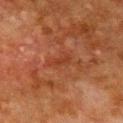Part of a total-body skin-imaging series; this lesion was reviewed on a skin check and was not flagged for biopsy. A 15 mm close-up extracted from a 3D total-body photography capture. On the chest. The subject is a male roughly 80 years of age.